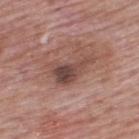Impression:
This lesion was catalogued during total-body skin photography and was not selected for biopsy.
Background:
From the upper back. A male subject, in their mid-60s. Imaged with white-light lighting. A 15 mm close-up extracted from a 3D total-body photography capture. The lesion's longest dimension is about 5.5 mm.